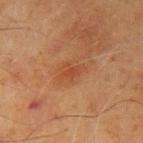workup: catalogued during a skin exam; not biopsied